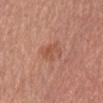Part of a total-body skin-imaging series; this lesion was reviewed on a skin check and was not flagged for biopsy. The lesion is on the abdomen. The patient is a male aged 63–67. A lesion tile, about 15 mm wide, cut from a 3D total-body photograph.